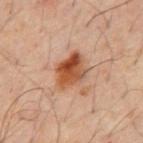– notes — imaged on a skin check; not biopsied
– automated lesion analysis — an area of roughly 11 mm² and a shape eccentricity near 0.7; a detector confidence of about 100 out of 100 that the crop contains a lesion
– subject — male, aged approximately 60
– image — ~15 mm crop, total-body skin-cancer survey
– size — ≈4.5 mm
– body site — the abdomen